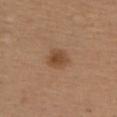Captured during whole-body skin photography for melanoma surveillance; the lesion was not biopsied. A female subject aged around 40. Located on the upper back. Cropped from a whole-body photographic skin survey; the tile spans about 15 mm.